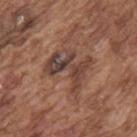Captured during whole-body skin photography for melanoma surveillance; the lesion was not biopsied.
A male subject aged around 75.
From the upper back.
A lesion tile, about 15 mm wide, cut from a 3D total-body photograph.